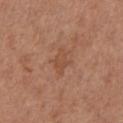Assessment: Imaged during a routine full-body skin examination; the lesion was not biopsied and no histopathology is available. Background: From the chest. A male subject, approximately 55 years of age. A roughly 15 mm field-of-view crop from a total-body skin photograph.